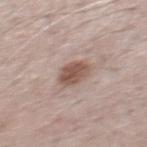{"biopsy_status": "not biopsied; imaged during a skin examination", "patient": {"sex": "male", "age_approx": 70}, "image": {"source": "total-body photography crop", "field_of_view_mm": 15}, "lighting": "white-light", "lesion_size": {"long_diameter_mm_approx": 3.0}, "site": "mid back"}